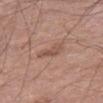Assessment:
The lesion was tiled from a total-body skin photograph and was not biopsied.